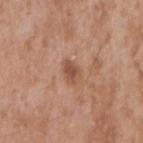<tbp_lesion>
  <biopsy_status>not biopsied; imaged during a skin examination</biopsy_status>
  <site>right upper arm</site>
  <lesion_size>
    <long_diameter_mm_approx>2.5</long_diameter_mm_approx>
  </lesion_size>
  <lighting>white-light</lighting>
  <patient>
    <sex>male</sex>
    <age_approx>50</age_approx>
  </patient>
  <image>
    <source>total-body photography crop</source>
    <field_of_view_mm>15</field_of_view_mm>
  </image>
  <automated_metrics>
    <area_mm2_approx>4.0</area_mm2_approx>
    <eccentricity>0.65</eccentricity>
    <shape_asymmetry>0.3</shape_asymmetry>
    <cielab_L>51</cielab_L>
    <cielab_a>22</cielab_a>
    <cielab_b>30</cielab_b>
    <vs_skin_darker_L>10.0</vs_skin_darker_L>
    <vs_skin_contrast_norm>7.0</vs_skin_contrast_norm>
    <border_irregularity_0_10>2.5</border_irregularity_0_10>
    <color_variation_0_10>1.5</color_variation_0_10>
  </automated_metrics>
</tbp_lesion>